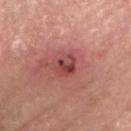This is a white-light tile. A male patient, aged 63 to 67. A region of skin cropped from a whole-body photographic capture, roughly 15 mm wide. On the head or neck. Histopathological examination showed a squamous cell carcinoma in situ (malignant).